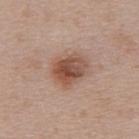notes: catalogued during a skin exam; not biopsied
imaging modality: total-body-photography crop, ~15 mm field of view
body site: the back
subject: female, aged 48 to 52
size: about 4 mm
TBP lesion metrics: an automated nevus-likeness rating near 95 out of 100 and a detector confidence of about 100 out of 100 that the crop contains a lesion